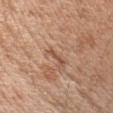workup: total-body-photography surveillance lesion; no biopsy
patient: female, aged 38–42
location: the left upper arm
illumination: white-light illumination
lesion diameter: ≈2.5 mm
acquisition: ~15 mm crop, total-body skin-cancer survey